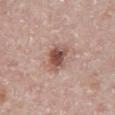Part of a total-body skin-imaging series; this lesion was reviewed on a skin check and was not flagged for biopsy.
A 15 mm close-up extracted from a 3D total-body photography capture.
The tile uses white-light illumination.
On the abdomen.
A male subject roughly 70 years of age.
Approximately 3.5 mm at its widest.